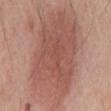follow-up = catalogued during a skin exam; not biopsied
body site = the mid back
size = ≈13.5 mm
patient = male, about 70 years old
automated metrics = roughly 10 lightness units darker than nearby skin; a border-irregularity index near 2.5/10, internal color variation of about 4 on a 0–10 scale, and peripheral color asymmetry of about 1
tile lighting = white-light
acquisition = ~15 mm crop, total-body skin-cancer survey